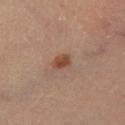Recorded during total-body skin imaging; not selected for excision or biopsy. Approximately 2.5 mm at its widest. On the leg. Automated tile analysis of the lesion measured a lesion area of about 4 mm², a shape eccentricity near 0.75, and two-axis asymmetry of about 0.25. It also reported a lesion–skin lightness drop of about 9 and a normalized lesion–skin contrast near 8. And it measured a lesion-detection confidence of about 100/100. The subject is a male aged around 60. A 15 mm close-up extracted from a 3D total-body photography capture. Captured under cross-polarized illumination.